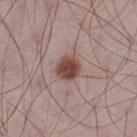The lesion was photographed on a routine skin check and not biopsied; there is no pathology result. The recorded lesion diameter is about 3 mm. From the left thigh. The subject is a male in their 70s. A 15 mm crop from a total-body photograph taken for skin-cancer surveillance. The lesion-visualizer software estimated a classifier nevus-likeness of about 100/100 and lesion-presence confidence of about 100/100.